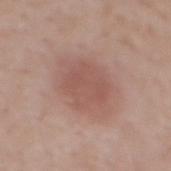Assessment: No biopsy was performed on this lesion — it was imaged during a full skin examination and was not determined to be concerning. Context: A male patient, aged 58 to 62. This is a white-light tile. The lesion is located on the mid back. A close-up tile cropped from a whole-body skin photograph, about 15 mm across.